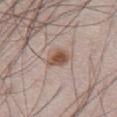The lesion was photographed on a routine skin check and not biopsied; there is no pathology result. A male subject approximately 70 years of age. Imaged with white-light lighting. A lesion tile, about 15 mm wide, cut from a 3D total-body photograph. The lesion is located on the abdomen. Automated tile analysis of the lesion measured a classifier nevus-likeness of about 95/100 and lesion-presence confidence of about 100/100.